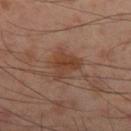{
  "biopsy_status": "not biopsied; imaged during a skin examination",
  "patient": {
    "sex": "male",
    "age_approx": 55
  },
  "site": "right thigh",
  "image": {
    "source": "total-body photography crop",
    "field_of_view_mm": 15
  }
}